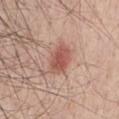Findings:
* follow-up · no biopsy performed (imaged during a skin exam)
* body site · the front of the torso
* image source · total-body-photography crop, ~15 mm field of view
* patient · male, aged approximately 40